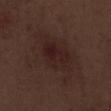This lesion was catalogued during total-body skin photography and was not selected for biopsy. Automated image analysis of the tile measured a border-irregularity rating of about 5/10, a within-lesion color-variation index near 4/10, and a peripheral color-asymmetry measure near 1. The analysis additionally found a detector confidence of about 100 out of 100 that the crop contains a lesion. A male subject, in their 70s. The lesion is on the right lower leg. Measured at roughly 8 mm in maximum diameter. A 15 mm close-up tile from a total-body photography series done for melanoma screening.